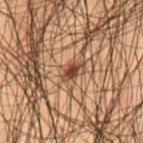* biopsy status — catalogued during a skin exam; not biopsied
* imaging modality — ~15 mm tile from a whole-body skin photo
* lesion size — ≈2.5 mm
* subject — male, aged around 50
* tile lighting — cross-polarized
* anatomic site — the lower back
* image-analysis metrics — a border-irregularity rating of about 3/10, a color-variation rating of about 4.5/10, and a peripheral color-asymmetry measure near 2